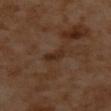Assessment:
Imaged during a routine full-body skin examination; the lesion was not biopsied and no histopathology is available.
Image and clinical context:
From the upper back. The patient is a female roughly 55 years of age. A 15 mm close-up extracted from a 3D total-body photography capture.